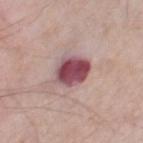The lesion was tiled from a total-body skin photograph and was not biopsied. From the right thigh. A male patient, aged approximately 70. A lesion tile, about 15 mm wide, cut from a 3D total-body photograph. Approximately 4 mm at its widest. Captured under white-light illumination.